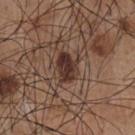Impression:
Part of a total-body skin-imaging series; this lesion was reviewed on a skin check and was not flagged for biopsy.
Clinical summary:
On the chest. The tile uses white-light illumination. A 15 mm close-up extracted from a 3D total-body photography capture. A male subject aged 53 to 57. Measured at roughly 3.5 mm in maximum diameter.